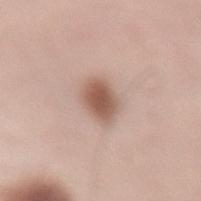Q: Lesion location?
A: the lower back
Q: Illumination type?
A: white-light
Q: What is the imaging modality?
A: total-body-photography crop, ~15 mm field of view
Q: Patient demographics?
A: female, roughly 50 years of age
Q: Lesion size?
A: ≈4 mm